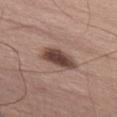Imaged during a routine full-body skin examination; the lesion was not biopsied and no histopathology is available.
A roughly 15 mm field-of-view crop from a total-body skin photograph.
The recorded lesion diameter is about 5 mm.
The subject is a male aged 63 to 67.
The lesion is located on the front of the torso.
The total-body-photography lesion software estimated an area of roughly 10 mm², an outline eccentricity of about 0.9 (0 = round, 1 = elongated), and a shape-asymmetry score of about 0.15 (0 = symmetric). The software also gave a mean CIELAB color near L≈45 a*≈18 b*≈23 and a lesion-to-skin contrast of about 10.5 (normalized; higher = more distinct). The analysis additionally found border irregularity of about 2 on a 0–10 scale, a within-lesion color-variation index near 5/10, and peripheral color asymmetry of about 1.5. It also reported an automated nevus-likeness rating near 90 out of 100.